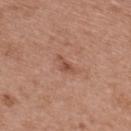  biopsy_status: not biopsied; imaged during a skin examination
  site: back
  patient:
    sex: female
    age_approx: 40
  lighting: white-light
  lesion_size:
    long_diameter_mm_approx: 2.5
  image:
    source: total-body photography crop
    field_of_view_mm: 15